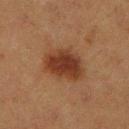Impression: Captured during whole-body skin photography for melanoma surveillance; the lesion was not biopsied. Acquisition and patient details: A female patient aged approximately 40. A 15 mm close-up tile from a total-body photography series done for melanoma screening. Automated tile analysis of the lesion measured a lesion area of about 15 mm², an eccentricity of roughly 0.7, and two-axis asymmetry of about 0.15. It also reported border irregularity of about 2 on a 0–10 scale, internal color variation of about 4 on a 0–10 scale, and a peripheral color-asymmetry measure near 1. Captured under cross-polarized illumination. Located on the right lower leg. Longest diameter approximately 5 mm.